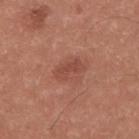Part of a total-body skin-imaging series; this lesion was reviewed on a skin check and was not flagged for biopsy. Located on the upper back. The tile uses white-light illumination. A lesion tile, about 15 mm wide, cut from a 3D total-body photograph. A male subject aged 23 to 27.